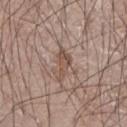Q: Was this lesion biopsied?
A: total-body-photography surveillance lesion; no biopsy
Q: How large is the lesion?
A: ~4 mm (longest diameter)
Q: What are the patient's age and sex?
A: male, about 75 years old
Q: What kind of image is this?
A: ~15 mm tile from a whole-body skin photo
Q: Automated lesion metrics?
A: a lesion area of about 4.5 mm², an eccentricity of roughly 0.9, and two-axis asymmetry of about 0.45
Q: Where on the body is the lesion?
A: the right forearm